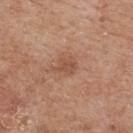Imaged during a routine full-body skin examination; the lesion was not biopsied and no histopathology is available. This is a white-light tile. A roughly 15 mm field-of-view crop from a total-body skin photograph. Longest diameter approximately 2.5 mm. On the back. A female subject aged around 75.